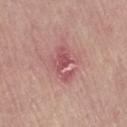Q: Was a biopsy performed?
A: catalogued during a skin exam; not biopsied
Q: Illumination type?
A: white-light
Q: Where on the body is the lesion?
A: the right thigh
Q: Lesion size?
A: about 4.5 mm
Q: How was this image acquired?
A: total-body-photography crop, ~15 mm field of view
Q: Patient demographics?
A: female, aged approximately 70